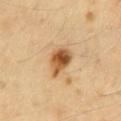Part of a total-body skin-imaging series; this lesion was reviewed on a skin check and was not flagged for biopsy.
A 15 mm crop from a total-body photograph taken for skin-cancer surveillance.
Imaged with cross-polarized lighting.
From the mid back.
About 3.5 mm across.
A male patient, aged approximately 40.
The total-body-photography lesion software estimated an automated nevus-likeness rating near 95 out of 100 and a detector confidence of about 100 out of 100 that the crop contains a lesion.